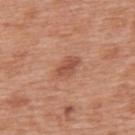| feature | finding |
|---|---|
| location | the upper back |
| image source | ~15 mm tile from a whole-body skin photo |
| subject | male, aged approximately 70 |
| diameter | ≈3.5 mm |